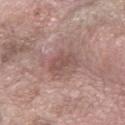Impression: The lesion was photographed on a routine skin check and not biopsied; there is no pathology result. Clinical summary: The tile uses white-light illumination. The lesion-visualizer software estimated a lesion area of about 6 mm², a shape eccentricity near 0.8, and a symmetry-axis asymmetry near 0.35. The software also gave a classifier nevus-likeness of about 0/100. A 15 mm close-up extracted from a 3D total-body photography capture. A male subject aged 58–62. The recorded lesion diameter is about 3.5 mm. The lesion is located on the left forearm.